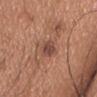{"biopsy_status": "not biopsied; imaged during a skin examination", "patient": {"sex": "male", "age_approx": 45}, "site": "chest", "image": {"source": "total-body photography crop", "field_of_view_mm": 15}}